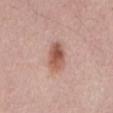workup = no biopsy performed (imaged during a skin exam) | anatomic site = the mid back | diameter = ≈4 mm | lighting = white-light illumination | image = ~15 mm tile from a whole-body skin photo | subject = male, in their mid-60s | image-analysis metrics = a nevus-likeness score of about 95/100.